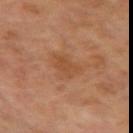Automated image analysis of the tile measured a mean CIELAB color near L≈46 a*≈21 b*≈34, a lesion–skin lightness drop of about 6, and a normalized border contrast of about 5. The analysis additionally found an automated nevus-likeness rating near 0 out of 100 and lesion-presence confidence of about 100/100. The recorded lesion diameter is about 3 mm. On the right upper arm. This image is a 15 mm lesion crop taken from a total-body photograph. A male subject aged 63–67.